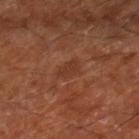- location · the right lower leg
- image-analysis metrics · a mean CIELAB color near L≈35 a*≈24 b*≈30, roughly 6 lightness units darker than nearby skin, and a normalized border contrast of about 5; a nevus-likeness score of about 0/100 and a detector confidence of about 100 out of 100 that the crop contains a lesion
- illumination · cross-polarized illumination
- lesion size · about 3 mm
- imaging modality · ~15 mm tile from a whole-body skin photo
- subject · approximately 65 years of age